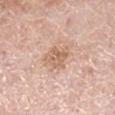biopsy status: imaged on a skin check; not biopsied
acquisition: 15 mm crop, total-body photography
subject: female, in their mid- to late 60s
body site: the right lower leg
tile lighting: white-light